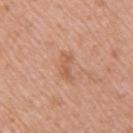| key | value |
|---|---|
| workup | no biopsy performed (imaged during a skin exam) |
| image source | ~15 mm tile from a whole-body skin photo |
| subject | female, aged 38 to 42 |
| lesion diameter | ~3.5 mm (longest diameter) |
| location | the right upper arm |
| lighting | white-light |